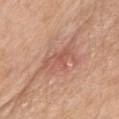The lesion was photographed on a routine skin check and not biopsied; there is no pathology result. The lesion is on the chest. A female subject in their 70s. A region of skin cropped from a whole-body photographic capture, roughly 15 mm wide.